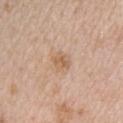Imaged during a routine full-body skin examination; the lesion was not biopsied and no histopathology is available.
This is a white-light tile.
The lesion's longest dimension is about 2.5 mm.
The total-body-photography lesion software estimated a mean CIELAB color near L≈61 a*≈18 b*≈33 and roughly 8 lightness units darker than nearby skin. The software also gave a within-lesion color-variation index near 3/10 and radial color variation of about 1.
A female subject in their mid- to late 40s.
Located on the upper back.
A roughly 15 mm field-of-view crop from a total-body skin photograph.